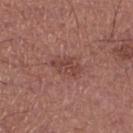<tbp_lesion>
  <biopsy_status>not biopsied; imaged during a skin examination</biopsy_status>
  <image>
    <source>total-body photography crop</source>
    <field_of_view_mm>15</field_of_view_mm>
  </image>
  <site>left thigh</site>
  <patient>
    <sex>male</sex>
    <age_approx>65</age_approx>
  </patient>
</tbp_lesion>